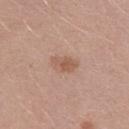biopsy_status: not biopsied; imaged during a skin examination